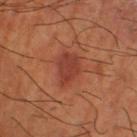This lesion was catalogued during total-body skin photography and was not selected for biopsy.
From the right lower leg.
The tile uses cross-polarized illumination.
The recorded lesion diameter is about 4 mm.
A male subject, approximately 65 years of age.
A close-up tile cropped from a whole-body skin photograph, about 15 mm across.